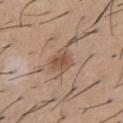Captured during whole-body skin photography for melanoma surveillance; the lesion was not biopsied.
Longest diameter approximately 3 mm.
A region of skin cropped from a whole-body photographic capture, roughly 15 mm wide.
The lesion-visualizer software estimated a lesion color around L≈52 a*≈18 b*≈30 in CIELAB. And it measured a classifier nevus-likeness of about 80/100 and lesion-presence confidence of about 100/100.
A male patient aged approximately 60.
Located on the chest.